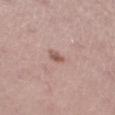The lesion was tiled from a total-body skin photograph and was not biopsied. Imaged with white-light lighting. Measured at roughly 3 mm in maximum diameter. Located on the leg. An algorithmic analysis of the crop reported a lesion area of about 5 mm² and a shape-asymmetry score of about 0.2 (0 = symmetric). Cropped from a total-body skin-imaging series; the visible field is about 15 mm. A female subject roughly 45 years of age.